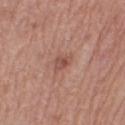The lesion was tiled from a total-body skin photograph and was not biopsied. Imaged with white-light lighting. A 15 mm close-up extracted from a 3D total-body photography capture. A female subject, about 55 years old. Longest diameter approximately 2.5 mm. On the right thigh.